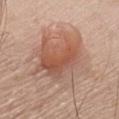The lesion was tiled from a total-body skin photograph and was not biopsied.
The subject is a male roughly 60 years of age.
A 15 mm crop from a total-body photograph taken for skin-cancer surveillance.
Longest diameter approximately 7 mm.
This is a white-light tile.
The lesion is on the chest.